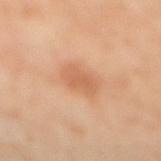<case>
  <biopsy_status>not biopsied; imaged during a skin examination</biopsy_status>
  <image>
    <source>total-body photography crop</source>
    <field_of_view_mm>15</field_of_view_mm>
  </image>
  <site>right lower leg</site>
  <patient>
    <sex>female</sex>
    <age_approx>50</age_approx>
  </patient>
  <lighting>cross-polarized</lighting>
</case>